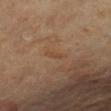Notes:
* follow-up · total-body-photography surveillance lesion; no biopsy
* image-analysis metrics · border irregularity of about 3.5 on a 0–10 scale and a within-lesion color-variation index near 0/10
* imaging modality · 15 mm crop, total-body photography
* lesion diameter · ≈2.5 mm
* body site · the right leg
* patient · female, roughly 65 years of age